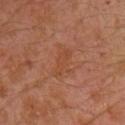notes: total-body-photography surveillance lesion; no biopsy | acquisition: total-body-photography crop, ~15 mm field of view | location: the left arm | subject: male, aged approximately 30 | diameter: about 4 mm | lighting: cross-polarized illumination.